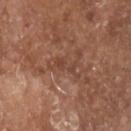Clinical impression: This lesion was catalogued during total-body skin photography and was not selected for biopsy. Background: A region of skin cropped from a whole-body photographic capture, roughly 15 mm wide. Approximately 3 mm at its widest. The lesion is on the head or neck. Captured under white-light illumination. The subject is a male aged 78–82.